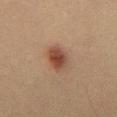Impression: Part of a total-body skin-imaging series; this lesion was reviewed on a skin check and was not flagged for biopsy. Acquisition and patient details: A male subject aged approximately 35. The lesion's longest dimension is about 4 mm. Imaged with cross-polarized lighting. A region of skin cropped from a whole-body photographic capture, roughly 15 mm wide. The lesion is located on the chest.